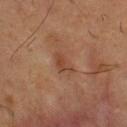{"site": "chest", "patient": {"sex": "male", "age_approx": 55}, "image": {"source": "total-body photography crop", "field_of_view_mm": 15}}